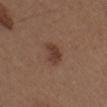Findings:
* diameter · ≈3 mm
* tile lighting · white-light
* subject · male, in their 30s
* image · 15 mm crop, total-body photography
* location · the right upper arm
* TBP lesion metrics · a border-irregularity index near 2.5/10, a color-variation rating of about 2.5/10, and a peripheral color-asymmetry measure near 0.5